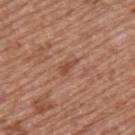The lesion was tiled from a total-body skin photograph and was not biopsied. A male subject, in their mid-50s. On the upper back. Captured under white-light illumination. A 15 mm crop from a total-body photograph taken for skin-cancer surveillance. About 2.5 mm across.